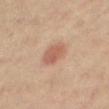Part of a total-body skin-imaging series; this lesion was reviewed on a skin check and was not flagged for biopsy. A 15 mm close-up tile from a total-body photography series done for melanoma screening. The lesion is on the right thigh. A male subject, roughly 60 years of age.